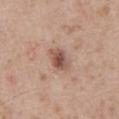Case summary:
* workup: no biopsy performed (imaged during a skin exam)
* subject: male, roughly 55 years of age
* automated lesion analysis: a mean CIELAB color near L≈52 a*≈21 b*≈26, about 13 CIELAB-L* units darker than the surrounding skin, and a lesion-to-skin contrast of about 8.5 (normalized; higher = more distinct)
* diameter: ≈3 mm
* tile lighting: white-light
* acquisition: ~15 mm crop, total-body skin-cancer survey
* location: the abdomen